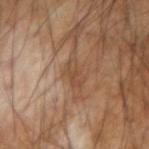biopsy status = no biopsy performed (imaged during a skin exam) | lighting = cross-polarized | lesion size = ≈3 mm | location = the right forearm | imaging modality = total-body-photography crop, ~15 mm field of view | patient = male, in their mid-50s.